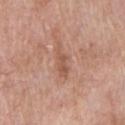Q: Is there a histopathology result?
A: catalogued during a skin exam; not biopsied
Q: Automated lesion metrics?
A: an area of roughly 5 mm², a shape eccentricity near 0.95, and a symmetry-axis asymmetry near 0.35; a within-lesion color-variation index near 1/10 and a peripheral color-asymmetry measure near 0
Q: What lighting was used for the tile?
A: white-light
Q: Patient demographics?
A: male, aged approximately 65
Q: Lesion size?
A: ~4.5 mm (longest diameter)
Q: Lesion location?
A: the chest
Q: How was this image acquired?
A: ~15 mm tile from a whole-body skin photo